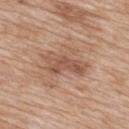imaging modality = total-body-photography crop, ~15 mm field of view | patient = female, aged approximately 40 | location = the upper back.